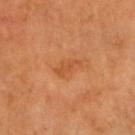Findings:
- follow-up · no biopsy performed (imaged during a skin exam)
- acquisition · ~15 mm crop, total-body skin-cancer survey
- illumination · cross-polarized
- image-analysis metrics · a color-variation rating of about 0.5/10 and a peripheral color-asymmetry measure near 0; an automated nevus-likeness rating near 0 out of 100 and lesion-presence confidence of about 100/100
- anatomic site · the head or neck
- patient · male, approximately 60 years of age